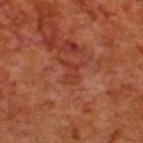Q: Is there a histopathology result?
A: catalogued during a skin exam; not biopsied
Q: What is the imaging modality?
A: ~15 mm tile from a whole-body skin photo
Q: Lesion size?
A: ~3 mm (longest diameter)
Q: Who is the patient?
A: male, aged 68 to 72
Q: How was the tile lit?
A: cross-polarized
Q: Lesion location?
A: the upper back